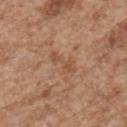notes: no biopsy performed (imaged during a skin exam) | subject: male, roughly 65 years of age | size: about 4 mm | body site: the upper back | image-analysis metrics: a lesion–skin lightness drop of about 7 and a lesion-to-skin contrast of about 5.5 (normalized; higher = more distinct); a classifier nevus-likeness of about 0/100 and a detector confidence of about 100 out of 100 that the crop contains a lesion | tile lighting: white-light illumination | imaging modality: ~15 mm tile from a whole-body skin photo.